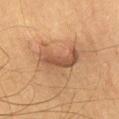Clinical impression: This lesion was catalogued during total-body skin photography and was not selected for biopsy. Context: A lesion tile, about 15 mm wide, cut from a 3D total-body photograph. The patient is a male approximately 65 years of age. The total-body-photography lesion software estimated a classifier nevus-likeness of about 65/100 and lesion-presence confidence of about 95/100. From the mid back. Imaged with cross-polarized lighting. The recorded lesion diameter is about 6 mm.